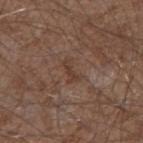biopsy_status: not biopsied; imaged during a skin examination
patient:
  sex: male
  age_approx: 75
lesion_size:
  long_diameter_mm_approx: 3.0
image:
  source: total-body photography crop
  field_of_view_mm: 15
site: right forearm
automated_metrics:
  area_mm2_approx: 3.0
  shape_asymmetry: 0.45
  cielab_L: 38
  cielab_a: 18
  cielab_b: 25
  vs_skin_darker_L: 6.0
  vs_skin_contrast_norm: 5.5
  lesion_detection_confidence_0_100: 95